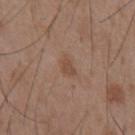Impression:
Recorded during total-body skin imaging; not selected for excision or biopsy.
Acquisition and patient details:
The lesion's longest dimension is about 2.5 mm. Automated tile analysis of the lesion measured a border-irregularity rating of about 3/10, a color-variation rating of about 1/10, and peripheral color asymmetry of about 0.5. The lesion is located on the abdomen. The subject is a male aged 53 to 57. This image is a 15 mm lesion crop taken from a total-body photograph.